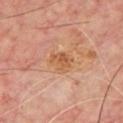Part of a total-body skin-imaging series; this lesion was reviewed on a skin check and was not flagged for biopsy.
Approximately 3 mm at its widest.
A male subject aged 63–67.
On the chest.
A 15 mm crop from a total-body photograph taken for skin-cancer surveillance.
This is a cross-polarized tile.